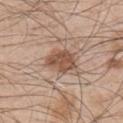Case summary:
* biopsy status: imaged on a skin check; not biopsied
* imaging modality: 15 mm crop, total-body photography
* body site: the right upper arm
* TBP lesion metrics: about 12 CIELAB-L* units darker than the surrounding skin and a normalized lesion–skin contrast near 9; border irregularity of about 3 on a 0–10 scale and peripheral color asymmetry of about 1; an automated nevus-likeness rating near 85 out of 100 and a lesion-detection confidence of about 100/100
* patient: male, aged 48–52